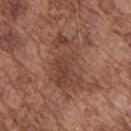– lighting · white-light
– location · the upper back
– subject · male, aged around 75
– TBP lesion metrics · a footprint of about 22 mm², an eccentricity of roughly 0.85, and a symmetry-axis asymmetry near 0.25; an average lesion color of about L≈43 a*≈21 b*≈26 (CIELAB) and roughly 8 lightness units darker than nearby skin; a border-irregularity index near 4.5/10, a within-lesion color-variation index near 4.5/10, and radial color variation of about 1.5
– image source · ~15 mm tile from a whole-body skin photo
– lesion size · ~8 mm (longest diameter)